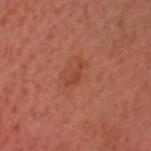Findings:
• biopsy status: no biopsy performed (imaged during a skin exam)
• anatomic site: the head or neck
• tile lighting: cross-polarized
• subject: male, in their mid-30s
• acquisition: 15 mm crop, total-body photography